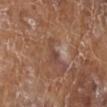On the leg.
The recorded lesion diameter is about 3 mm.
Captured under white-light illumination.
Automated image analysis of the tile measured a nevus-likeness score of about 0/100 and lesion-presence confidence of about 95/100.
A roughly 15 mm field-of-view crop from a total-body skin photograph.
The subject is a female aged approximately 75.